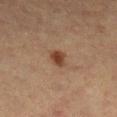follow-up — no biopsy performed (imaged during a skin exam); subject — female, aged 58 to 62; TBP lesion metrics — a nevus-likeness score of about 100/100 and a lesion-detection confidence of about 100/100; diameter — about 2.5 mm; illumination — cross-polarized illumination; imaging modality — ~15 mm tile from a whole-body skin photo; site — the right lower leg.